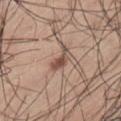notes=imaged on a skin check; not biopsied
lighting=white-light
automated lesion analysis=an average lesion color of about L≈51 a*≈18 b*≈25 (CIELAB), about 12 CIELAB-L* units darker than the surrounding skin, and a normalized lesion–skin contrast near 8.5; a border-irregularity index near 5/10, a within-lesion color-variation index near 3/10, and peripheral color asymmetry of about 1
size=~3.5 mm (longest diameter)
body site=the left thigh
acquisition=~15 mm tile from a whole-body skin photo
subject=male, in their mid-50s